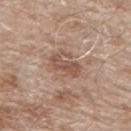workup: total-body-photography surveillance lesion; no biopsy | subject: male, aged 78–82 | tile lighting: white-light | diameter: about 4 mm | location: the back | imaging modality: ~15 mm crop, total-body skin-cancer survey.